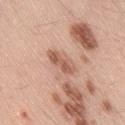notes: imaged on a skin check; not biopsied | acquisition: ~15 mm crop, total-body skin-cancer survey | image-analysis metrics: internal color variation of about 3 on a 0–10 scale and radial color variation of about 1 | location: the leg | diameter: ~4 mm (longest diameter) | patient: male, aged approximately 55 | illumination: white-light.